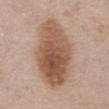image:
  source: total-body photography crop
  field_of_view_mm: 15
site: abdomen
patient:
  sex: male
  age_approx: 75